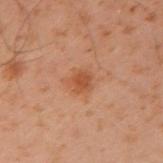Case summary:
– notes — catalogued during a skin exam; not biopsied
– anatomic site — the arm
– lesion diameter — ≈2.5 mm
– patient — male, aged around 50
– automated metrics — a nevus-likeness score of about 35/100 and a lesion-detection confidence of about 100/100
– illumination — cross-polarized
– image source — 15 mm crop, total-body photography